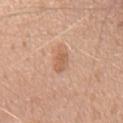workup = total-body-photography surveillance lesion; no biopsy | automated metrics = internal color variation of about 2.5 on a 0–10 scale and a peripheral color-asymmetry measure near 1; a nevus-likeness score of about 45/100 and lesion-presence confidence of about 100/100 | lighting = white-light | acquisition = ~15 mm crop, total-body skin-cancer survey | patient = male, in their mid-60s | size = ~3 mm (longest diameter) | anatomic site = the upper back.